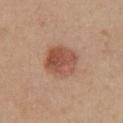| feature | finding |
|---|---|
| notes | imaged on a skin check; not biopsied |
| patient | female, aged around 45 |
| body site | the chest |
| image | total-body-photography crop, ~15 mm field of view |